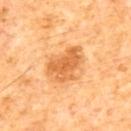notes: total-body-photography surveillance lesion; no biopsy
TBP lesion metrics: a lesion area of about 13 mm², a shape eccentricity near 0.75, and a symmetry-axis asymmetry near 0.3; a classifier nevus-likeness of about 60/100 and a lesion-detection confidence of about 100/100
image: ~15 mm crop, total-body skin-cancer survey
anatomic site: the mid back
subject: male, aged approximately 65
tile lighting: cross-polarized
size: ≈5 mm